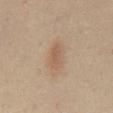Notes:
* subject: male, aged approximately 50
* image: 15 mm crop, total-body photography
* automated lesion analysis: a mean CIELAB color near L≈50 a*≈15 b*≈27, a lesion–skin lightness drop of about 7, and a normalized lesion–skin contrast near 5.5; an automated nevus-likeness rating near 60 out of 100
* anatomic site: the chest
* illumination: cross-polarized